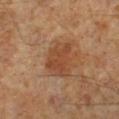Assessment:
Part of a total-body skin-imaging series; this lesion was reviewed on a skin check and was not flagged for biopsy.
Acquisition and patient details:
Measured at roughly 4 mm in maximum diameter. An algorithmic analysis of the crop reported an average lesion color of about L≈42 a*≈21 b*≈32 (CIELAB), about 8 CIELAB-L* units darker than the surrounding skin, and a lesion-to-skin contrast of about 6.5 (normalized; higher = more distinct). The software also gave a border-irregularity index near 2.5/10 and a within-lesion color-variation index near 3/10. It also reported an automated nevus-likeness rating near 55 out of 100 and lesion-presence confidence of about 100/100. A male subject aged approximately 60. A 15 mm crop from a total-body photograph taken for skin-cancer surveillance. Located on the left lower leg. This is a cross-polarized tile.